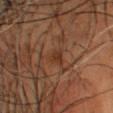Part of a total-body skin-imaging series; this lesion was reviewed on a skin check and was not flagged for biopsy. A male subject aged approximately 55. A close-up tile cropped from a whole-body skin photograph, about 15 mm across. The lesion-visualizer software estimated a lesion area of about 3.5 mm² and an eccentricity of roughly 0.9. It also reported a lesion color around L≈28 a*≈17 b*≈25 in CIELAB, about 5 CIELAB-L* units darker than the surrounding skin, and a normalized border contrast of about 6. The analysis additionally found a border-irregularity rating of about 3.5/10, a color-variation rating of about 1.5/10, and radial color variation of about 0.5. It also reported lesion-presence confidence of about 85/100. The lesion is located on the head or neck. Measured at roughly 3 mm in maximum diameter.